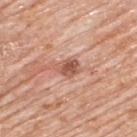The lesion was photographed on a routine skin check and not biopsied; there is no pathology result. The total-body-photography lesion software estimated border irregularity of about 2.5 on a 0–10 scale and radial color variation of about 1. The analysis additionally found a classifier nevus-likeness of about 5/100 and a detector confidence of about 100 out of 100 that the crop contains a lesion. A 15 mm close-up extracted from a 3D total-body photography capture. From the upper back. The recorded lesion diameter is about 2.5 mm. The subject is a male aged 78–82.